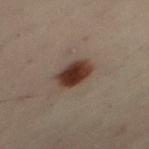From the left thigh.
This image is a 15 mm lesion crop taken from a total-body photograph.
A female patient, aged approximately 50.